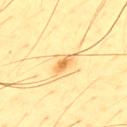Captured during whole-body skin photography for melanoma surveillance; the lesion was not biopsied.
Automated tile analysis of the lesion measured a shape-asymmetry score of about 0.25 (0 = symmetric). It also reported an automated nevus-likeness rating near 85 out of 100 and lesion-presence confidence of about 100/100.
On the upper back.
The subject is a male aged approximately 45.
Approximately 3 mm at its widest.
Cropped from a whole-body photographic skin survey; the tile spans about 15 mm.